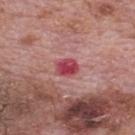Q: Was a biopsy performed?
A: total-body-photography surveillance lesion; no biopsy
Q: How large is the lesion?
A: ≈2.5 mm
Q: What kind of image is this?
A: total-body-photography crop, ~15 mm field of view
Q: Who is the patient?
A: male, aged approximately 70
Q: Illumination type?
A: white-light
Q: Automated lesion metrics?
A: an eccentricity of roughly 0.6; a border-irregularity rating of about 1.5/10 and peripheral color asymmetry of about 1; a nevus-likeness score of about 0/100 and a lesion-detection confidence of about 100/100
Q: What is the anatomic site?
A: the mid back